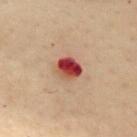Recorded during total-body skin imaging; not selected for excision or biopsy.
Located on the chest.
A roughly 15 mm field-of-view crop from a total-body skin photograph.
The tile uses cross-polarized illumination.
The patient is a female roughly 50 years of age.